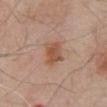Captured under white-light illumination. Cropped from a whole-body photographic skin survey; the tile spans about 15 mm. The subject is a male about 80 years old. Approximately 3.5 mm at its widest. Automated tile analysis of the lesion measured a lesion color around L≈53 a*≈20 b*≈31 in CIELAB, roughly 9 lightness units darker than nearby skin, and a normalized lesion–skin contrast near 7.5. The analysis additionally found an automated nevus-likeness rating near 75 out of 100 and a lesion-detection confidence of about 100/100. The lesion is located on the abdomen.